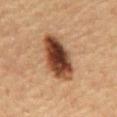Recorded during total-body skin imaging; not selected for excision or biopsy.
A male patient, aged around 65.
Longest diameter approximately 6 mm.
Imaged with cross-polarized lighting.
A lesion tile, about 15 mm wide, cut from a 3D total-body photograph.
The lesion is on the back.